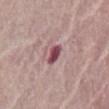{
  "biopsy_status": "not biopsied; imaged during a skin examination",
  "lesion_size": {
    "long_diameter_mm_approx": 2.5
  },
  "patient": {
    "sex": "female",
    "age_approx": 65
  },
  "image": {
    "source": "total-body photography crop",
    "field_of_view_mm": 15
  },
  "automated_metrics": {
    "eccentricity": 0.75,
    "shape_asymmetry": 0.2,
    "cielab_L": 47,
    "cielab_a": 27,
    "cielab_b": 15,
    "vs_skin_darker_L": 16.0,
    "border_irregularity_0_10": 2.0,
    "color_variation_0_10": 3.0,
    "peripheral_color_asymmetry": 1.0
  },
  "site": "abdomen"
}